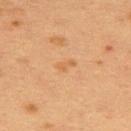Q: Is there a histopathology result?
A: imaged on a skin check; not biopsied
Q: What lighting was used for the tile?
A: cross-polarized
Q: What is the anatomic site?
A: the back
Q: What is the imaging modality?
A: ~15 mm tile from a whole-body skin photo
Q: How large is the lesion?
A: about 2.5 mm
Q: Patient demographics?
A: female, in their 40s
Q: What did automated image analysis measure?
A: border irregularity of about 2.5 on a 0–10 scale, a within-lesion color-variation index near 1/10, and a peripheral color-asymmetry measure near 0